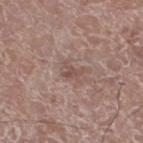Part of a total-body skin-imaging series; this lesion was reviewed on a skin check and was not flagged for biopsy.
This is a white-light tile.
On the left lower leg.
A male patient, in their mid-70s.
The lesion-visualizer software estimated about 8 CIELAB-L* units darker than the surrounding skin. The software also gave a detector confidence of about 100 out of 100 that the crop contains a lesion.
A region of skin cropped from a whole-body photographic capture, roughly 15 mm wide.